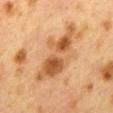Assessment: Captured during whole-body skin photography for melanoma surveillance; the lesion was not biopsied. Image and clinical context: This is a cross-polarized tile. On the mid back. A female subject, about 40 years old. This image is a 15 mm lesion crop taken from a total-body photograph.